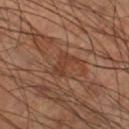Findings:
• follow-up · catalogued during a skin exam; not biopsied
• automated lesion analysis · a lesion area of about 5 mm² and a shape-asymmetry score of about 0.4 (0 = symmetric); a lesion color around L≈38 a*≈21 b*≈29 in CIELAB, roughly 6 lightness units darker than nearby skin, and a lesion-to-skin contrast of about 5.5 (normalized; higher = more distinct); a border-irregularity index near 5/10, internal color variation of about 2 on a 0–10 scale, and peripheral color asymmetry of about 0.5; a nevus-likeness score of about 0/100 and a detector confidence of about 50 out of 100 that the crop contains a lesion
• subject · male, aged 58–62
• imaging modality · ~15 mm tile from a whole-body skin photo
• anatomic site · the right lower leg
• tile lighting · cross-polarized